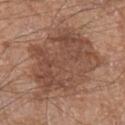Q: Was this lesion biopsied?
A: catalogued during a skin exam; not biopsied
Q: How was this image acquired?
A: total-body-photography crop, ~15 mm field of view
Q: What are the patient's age and sex?
A: male, about 65 years old
Q: How large is the lesion?
A: ≈10 mm
Q: Illumination type?
A: white-light illumination
Q: Where on the body is the lesion?
A: the left forearm